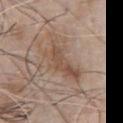Assessment: No biopsy was performed on this lesion — it was imaged during a full skin examination and was not determined to be concerning. Clinical summary: Measured at roughly 7 mm in maximum diameter. The lesion is on the chest. A 15 mm close-up tile from a total-body photography series done for melanoma screening. The total-body-photography lesion software estimated a border-irregularity index near 6.5/10, a color-variation rating of about 4/10, and radial color variation of about 1.5. The subject is a male approximately 80 years of age.